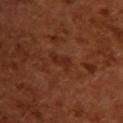Assessment:
The lesion was photographed on a routine skin check and not biopsied; there is no pathology result.
Image and clinical context:
Captured under cross-polarized illumination. An algorithmic analysis of the crop reported an area of roughly 3.5 mm², an eccentricity of roughly 0.9, and a shape-asymmetry score of about 0.4 (0 = symmetric). The software also gave an average lesion color of about L≈28 a*≈25 b*≈30 (CIELAB) and a normalized border contrast of about 5.5. The analysis additionally found a nevus-likeness score of about 0/100. The lesion is on the back. A lesion tile, about 15 mm wide, cut from a 3D total-body photograph. A female patient, aged around 55. Measured at roughly 3 mm in maximum diameter.